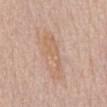No biopsy was performed on this lesion — it was imaged during a full skin examination and was not determined to be concerning. A female patient approximately 65 years of age. A 15 mm close-up tile from a total-body photography series done for melanoma screening. Automated tile analysis of the lesion measured border irregularity of about 6.5 on a 0–10 scale. It also reported a nevus-likeness score of about 0/100 and a lesion-detection confidence of about 100/100. Measured at roughly 6 mm in maximum diameter. The lesion is on the chest.